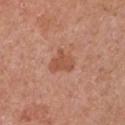notes: total-body-photography surveillance lesion; no biopsy
acquisition: 15 mm crop, total-body photography
subject: female, about 50 years old
location: the chest